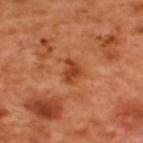This lesion was catalogued during total-body skin photography and was not selected for biopsy. Imaged with cross-polarized lighting. A 15 mm close-up tile from a total-body photography series done for melanoma screening. The lesion is on the upper back. Approximately 2.5 mm at its widest. A male subject, aged approximately 50.